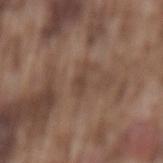A region of skin cropped from a whole-body photographic capture, roughly 15 mm wide. The patient is a male approximately 75 years of age. The total-body-photography lesion software estimated a nevus-likeness score of about 0/100 and lesion-presence confidence of about 95/100. The lesion is located on the mid back.